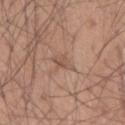Q: Was this lesion biopsied?
A: no biopsy performed (imaged during a skin exam)
Q: Patient demographics?
A: male, aged 53 to 57
Q: What did automated image analysis measure?
A: a footprint of about 3.5 mm², an eccentricity of roughly 0.9, and a symmetry-axis asymmetry near 0.45; a lesion color around L≈53 a*≈18 b*≈27 in CIELAB, about 8 CIELAB-L* units darker than the surrounding skin, and a normalized lesion–skin contrast near 5.5; a classifier nevus-likeness of about 0/100
Q: What is the anatomic site?
A: the left forearm
Q: What kind of image is this?
A: ~15 mm tile from a whole-body skin photo
Q: Illumination type?
A: white-light